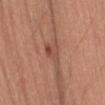The lesion was photographed on a routine skin check and not biopsied; there is no pathology result.
A female subject, aged 48–52.
The lesion is on the leg.
The tile uses white-light illumination.
A 15 mm crop from a total-body photograph taken for skin-cancer surveillance.